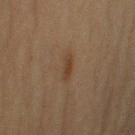Findings:
• follow-up · no biopsy performed (imaged during a skin exam)
• subject · female, aged 63–67
• image · ~15 mm tile from a whole-body skin photo
• anatomic site · the right upper arm
• diameter · ~3 mm (longest diameter)
• lighting · cross-polarized